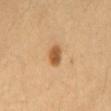Case summary:
– workup: total-body-photography surveillance lesion; no biopsy
– location: the abdomen
– subject: female, aged around 40
– image source: total-body-photography crop, ~15 mm field of view